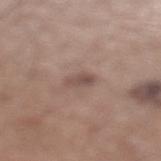biopsy status — catalogued during a skin exam; not biopsied
acquisition — ~15 mm crop, total-body skin-cancer survey
site — the left lower leg
automated lesion analysis — an area of roughly 3.5 mm², an outline eccentricity of about 0.8 (0 = round, 1 = elongated), and a shape-asymmetry score of about 0.2 (0 = symmetric); an average lesion color of about L≈49 a*≈16 b*≈22 (CIELAB); border irregularity of about 2 on a 0–10 scale and radial color variation of about 0.5
subject — male, aged 63 to 67
lighting — white-light illumination